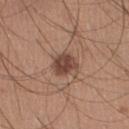This lesion was catalogued during total-body skin photography and was not selected for biopsy. A 15 mm close-up tile from a total-body photography series done for melanoma screening. From the right lower leg. A male patient about 30 years old. Captured under white-light illumination.